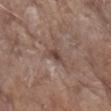No biopsy was performed on this lesion — it was imaged during a full skin examination and was not determined to be concerning. This is a white-light tile. On the left forearm. A 15 mm close-up extracted from a 3D total-body photography capture. The subject is a female in their mid-80s or older. Longest diameter approximately 2.5 mm.